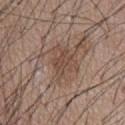The lesion is on the chest. Imaged with white-light lighting. A close-up tile cropped from a whole-body skin photograph, about 15 mm across. Automated image analysis of the tile measured a border-irregularity index near 4/10 and radial color variation of about 0.5. The software also gave a detector confidence of about 90 out of 100 that the crop contains a lesion. A male patient in their mid-50s.